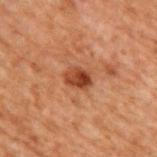notes: catalogued during a skin exam; not biopsied
patient: male, aged 68–72
tile lighting: cross-polarized illumination
acquisition: 15 mm crop, total-body photography
image-analysis metrics: an outline eccentricity of about 0.65 (0 = round, 1 = elongated)
anatomic site: the upper back
size: ≈3 mm